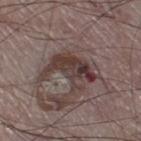Recorded during total-body skin imaging; not selected for excision or biopsy. A lesion tile, about 15 mm wide, cut from a 3D total-body photograph. Imaged with white-light lighting. On the right thigh. The lesion's longest dimension is about 6 mm. A male subject, aged 73–77.